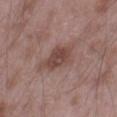Impression: Imaged during a routine full-body skin examination; the lesion was not biopsied and no histopathology is available. Background: A male subject aged 48–52. Located on the leg. A 15 mm close-up extracted from a 3D total-body photography capture.